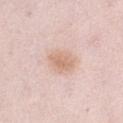Assessment:
Imaged during a routine full-body skin examination; the lesion was not biopsied and no histopathology is available.
Context:
The subject is a female roughly 65 years of age. The lesion is located on the front of the torso. Measured at roughly 3.5 mm in maximum diameter. The tile uses white-light illumination. A 15 mm close-up extracted from a 3D total-body photography capture.